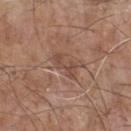Context: Approximately 3.5 mm at its widest. A 15 mm close-up extracted from a 3D total-body photography capture. From the chest. A male patient about 70 years old.